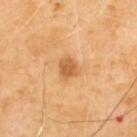- tile lighting — cross-polarized
- location — the upper back
- patient — male, aged 68 to 72
- imaging modality — 15 mm crop, total-body photography
- size — about 2.5 mm